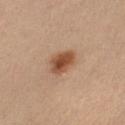Clinical impression:
The lesion was tiled from a total-body skin photograph and was not biopsied.
Context:
A lesion tile, about 15 mm wide, cut from a 3D total-body photograph. Approximately 4 mm at its widest. The lesion is on the left lower leg. This is a cross-polarized tile. A female subject in their 40s.